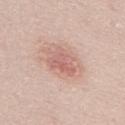Imaged during a routine full-body skin examination; the lesion was not biopsied and no histopathology is available.
The lesion is on the mid back.
A female patient, aged approximately 50.
Cropped from a total-body skin-imaging series; the visible field is about 15 mm.